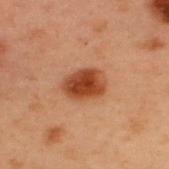notes — imaged on a skin check; not biopsied
lesion diameter — about 4.5 mm
illumination — cross-polarized
acquisition — total-body-photography crop, ~15 mm field of view
site — the upper back
subject — male, aged approximately 55
automated lesion analysis — a mean CIELAB color near L≈37 a*≈24 b*≈31 and about 13 CIELAB-L* units darker than the surrounding skin; a border-irregularity index near 1.5/10